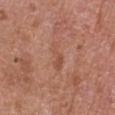{
  "biopsy_status": "not biopsied; imaged during a skin examination",
  "automated_metrics": {
    "area_mm2_approx": 4.5,
    "shape_asymmetry": 0.4,
    "cielab_L": 52,
    "cielab_a": 23,
    "cielab_b": 31,
    "vs_skin_darker_L": 6.0,
    "vs_skin_contrast_norm": 4.5
  },
  "lighting": "white-light",
  "patient": {
    "sex": "male",
    "age_approx": 65
  },
  "lesion_size": {
    "long_diameter_mm_approx": 4.0
  },
  "site": "chest",
  "image": {
    "source": "total-body photography crop",
    "field_of_view_mm": 15
  }
}